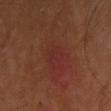notes: imaged on a skin check; not biopsied
illumination: cross-polarized
anatomic site: the chest
acquisition: total-body-photography crop, ~15 mm field of view
patient: male, aged 38 to 42